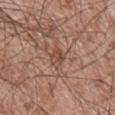No biopsy was performed on this lesion — it was imaged during a full skin examination and was not determined to be concerning.
The tile uses white-light illumination.
A lesion tile, about 15 mm wide, cut from a 3D total-body photograph.
Located on the mid back.
A male patient aged 53 to 57.
The lesion's longest dimension is about 3 mm.